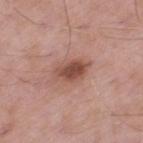This lesion was catalogued during total-body skin photography and was not selected for biopsy. Measured at roughly 4 mm in maximum diameter. The total-body-photography lesion software estimated a within-lesion color-variation index near 4.5/10. Cropped from a whole-body photographic skin survey; the tile spans about 15 mm. The tile uses white-light illumination. Located on the left thigh. A male patient, roughly 55 years of age.